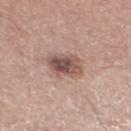location: the left lower leg | lesion diameter: ~4 mm (longest diameter) | patient: male, about 50 years old | automated lesion analysis: an area of roughly 9 mm², an outline eccentricity of about 0.7 (0 = round, 1 = elongated), and two-axis asymmetry of about 0.2; a border-irregularity rating of about 2/10 and a peripheral color-asymmetry measure near 2; an automated nevus-likeness rating near 70 out of 100 | image: ~15 mm tile from a whole-body skin photo | lighting: white-light illumination.